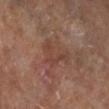Q: Is there a histopathology result?
A: total-body-photography surveillance lesion; no biopsy
Q: Lesion location?
A: the leg
Q: How was this image acquired?
A: total-body-photography crop, ~15 mm field of view
Q: What are the patient's age and sex?
A: female, about 75 years old